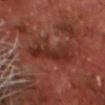A male subject about 70 years old. The lesion is located on the head or neck. Approximately 7 mm at its widest. The tile uses cross-polarized illumination. A 15 mm close-up extracted from a 3D total-body photography capture. Automated tile analysis of the lesion measured border irregularity of about 5.5 on a 0–10 scale, internal color variation of about 4.5 on a 0–10 scale, and a peripheral color-asymmetry measure near 1.5. The software also gave a classifier nevus-likeness of about 0/100 and a detector confidence of about 75 out of 100 that the crop contains a lesion.